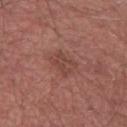Recorded during total-body skin imaging; not selected for excision or biopsy. The patient is a male aged approximately 55. From the leg. A lesion tile, about 15 mm wide, cut from a 3D total-body photograph.